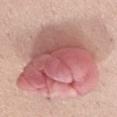Findings:
• automated metrics · a lesion area of about 65 mm² and a symmetry-axis asymmetry near 0.2; a mean CIELAB color near L≈58 a*≈27 b*≈23 and a normalized border contrast of about 9; border irregularity of about 2.5 on a 0–10 scale, a within-lesion color-variation index near 9.5/10, and peripheral color asymmetry of about 3
• location · the mid back
• subject · male, in their 50s
• image · ~15 mm crop, total-body skin-cancer survey